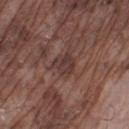Recorded during total-body skin imaging; not selected for excision or biopsy.
A lesion tile, about 15 mm wide, cut from a 3D total-body photograph.
A male subject, aged approximately 75.
The lesion is on the right lower leg.
The lesion's longest dimension is about 3 mm.